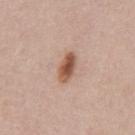workup = total-body-photography surveillance lesion; no biopsy
lighting = white-light
acquisition = 15 mm crop, total-body photography
body site = the abdomen
diameter = about 3.5 mm
subject = male, aged around 60